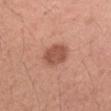<tbp_lesion>
<biopsy_status>not biopsied; imaged during a skin examination</biopsy_status>
<site>right forearm</site>
<image>
  <source>total-body photography crop</source>
  <field_of_view_mm>15</field_of_view_mm>
</image>
<lesion_size>
  <long_diameter_mm_approx>3.5</long_diameter_mm_approx>
</lesion_size>
<patient>
  <sex>female</sex>
  <age_approx>40</age_approx>
</patient>
<automated_metrics>
  <area_mm2_approx>8.5</area_mm2_approx>
  <shape_asymmetry>0.15</shape_asymmetry>
  <border_irregularity_0_10>1.0</border_irregularity_0_10>
  <color_variation_0_10>2.5</color_variation_0_10>
  <peripheral_color_asymmetry>1.0</peripheral_color_asymmetry>
</automated_metrics>
</tbp_lesion>